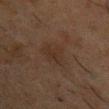The lesion was tiled from a total-body skin photograph and was not biopsied. A male patient aged 48–52. Cropped from a total-body skin-imaging series; the visible field is about 15 mm. From the left upper arm. The tile uses cross-polarized illumination.